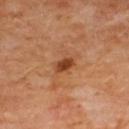Assessment:
Captured during whole-body skin photography for melanoma surveillance; the lesion was not biopsied.
Image and clinical context:
A roughly 15 mm field-of-view crop from a total-body skin photograph. The lesion-visualizer software estimated a lesion color around L≈42 a*≈26 b*≈36 in CIELAB and a normalized border contrast of about 9.5. It also reported a border-irregularity rating of about 2.5/10 and peripheral color asymmetry of about 0.5. The lesion is located on the upper back. Imaged with cross-polarized lighting. A female subject, in their 50s.